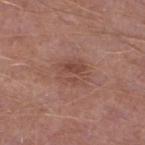image source: ~15 mm tile from a whole-body skin photo; illumination: white-light; patient: male, in their mid- to late 50s; site: the left lower leg; lesion size: about 4 mm.